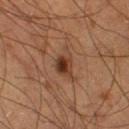Case summary:
* notes: imaged on a skin check; not biopsied
* lesion diameter: ~4 mm (longest diameter)
* image source: total-body-photography crop, ~15 mm field of view
* TBP lesion metrics: an average lesion color of about L≈38 a*≈21 b*≈30 (CIELAB), roughly 11 lightness units darker than nearby skin, and a normalized border contrast of about 9; a within-lesion color-variation index near 7/10 and peripheral color asymmetry of about 2.5; an automated nevus-likeness rating near 50 out of 100 and a lesion-detection confidence of about 100/100
* site: the right thigh
* patient: male, roughly 60 years of age
* illumination: cross-polarized